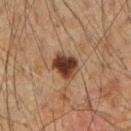biopsy status = no biopsy performed (imaged during a skin exam)
patient = male, about 50 years old
lesion size = about 3.5 mm
image source = ~15 mm tile from a whole-body skin photo
tile lighting = cross-polarized illumination
body site = the arm